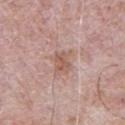follow-up: no biopsy performed (imaged during a skin exam)
lesion size: ~3 mm (longest diameter)
illumination: white-light
image source: 15 mm crop, total-body photography
patient: male, aged approximately 55